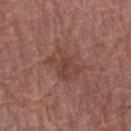workup: no biopsy performed (imaged during a skin exam)
location: the right forearm
subject: male, roughly 75 years of age
acquisition: 15 mm crop, total-body photography
lighting: white-light illumination